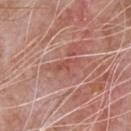<lesion>
  <biopsy_status>not biopsied; imaged during a skin examination</biopsy_status>
  <image>
    <source>total-body photography crop</source>
    <field_of_view_mm>15</field_of_view_mm>
  </image>
  <lighting>white-light</lighting>
  <automated_metrics>
    <cielab_L>52</cielab_L>
    <cielab_a>26</cielab_a>
    <cielab_b>28</cielab_b>
    <vs_skin_contrast_norm>6.0</vs_skin_contrast_norm>
    <border_irregularity_0_10>4.0</border_irregularity_0_10>
    <color_variation_0_10>0.5</color_variation_0_10>
    <peripheral_color_asymmetry>0.0</peripheral_color_asymmetry>
    <nevus_likeness_0_100>0</nevus_likeness_0_100>
    <lesion_detection_confidence_0_100>60</lesion_detection_confidence_0_100>
  </automated_metrics>
  <patient>
    <sex>male</sex>
    <age_approx>65</age_approx>
  </patient>
  <site>chest</site>
  <lesion_size>
    <long_diameter_mm_approx>3.5</long_diameter_mm_approx>
  </lesion_size>
</lesion>